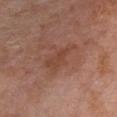Part of a total-body skin-imaging series; this lesion was reviewed on a skin check and was not flagged for biopsy. A 15 mm close-up extracted from a 3D total-body photography capture. The patient is a male in their 60s. On the chest. Captured under white-light illumination. Approximately 4 mm at its widest.